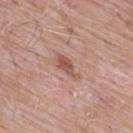Findings:
• notes: total-body-photography surveillance lesion; no biopsy
• patient: male, aged 63–67
• lesion size: ≈4 mm
• tile lighting: white-light
• body site: the back
• TBP lesion metrics: a lesion area of about 5 mm² and an outline eccentricity of about 0.9 (0 = round, 1 = elongated); an average lesion color of about L≈54 a*≈21 b*≈27 (CIELAB), about 10 CIELAB-L* units darker than the surrounding skin, and a lesion-to-skin contrast of about 7 (normalized; higher = more distinct); border irregularity of about 3.5 on a 0–10 scale, a within-lesion color-variation index near 1.5/10, and radial color variation of about 0.5
• acquisition: ~15 mm tile from a whole-body skin photo